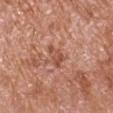Impression:
This lesion was catalogued during total-body skin photography and was not selected for biopsy.
Clinical summary:
A male patient about 65 years old. The tile uses white-light illumination. A region of skin cropped from a whole-body photographic capture, roughly 15 mm wide. On the left upper arm. The lesion-visualizer software estimated a lesion color around L≈52 a*≈25 b*≈31 in CIELAB, about 9 CIELAB-L* units darker than the surrounding skin, and a normalized lesion–skin contrast near 6.5. The analysis additionally found a border-irregularity rating of about 4.5/10 and a within-lesion color-variation index near 2.5/10. The analysis additionally found a classifier nevus-likeness of about 0/100. About 3.5 mm across.